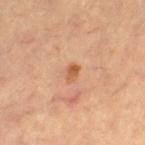Notes:
* notes: total-body-photography surveillance lesion; no biopsy
* site: the leg
* subject: female, aged around 65
* image source: ~15 mm tile from a whole-body skin photo